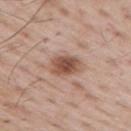This lesion was catalogued during total-body skin photography and was not selected for biopsy.
The patient is a male approximately 65 years of age.
On the upper back.
A 15 mm crop from a total-body photograph taken for skin-cancer surveillance.
The lesion-visualizer software estimated peripheral color asymmetry of about 1. It also reported an automated nevus-likeness rating near 95 out of 100 and a detector confidence of about 100 out of 100 that the crop contains a lesion.